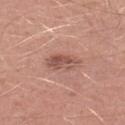Recorded during total-body skin imaging; not selected for excision or biopsy. This is a white-light tile. The lesion is on the leg. Automated tile analysis of the lesion measured an area of roughly 6.5 mm², an eccentricity of roughly 0.85, and two-axis asymmetry of about 0.25. The analysis additionally found a border-irregularity index near 3/10 and radial color variation of about 1.5. It also reported an automated nevus-likeness rating near 20 out of 100 and lesion-presence confidence of about 100/100. The patient is a male in their mid- to late 40s. The lesion's longest dimension is about 4 mm. A lesion tile, about 15 mm wide, cut from a 3D total-body photograph.